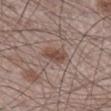<record>
  <biopsy_status>not biopsied; imaged during a skin examination</biopsy_status>
  <automated_metrics>
    <area_mm2_approx>5.0</area_mm2_approx>
    <eccentricity>0.75</eccentricity>
    <shape_asymmetry>0.2</shape_asymmetry>
    <border_irregularity_0_10>2.0</border_irregularity_0_10>
    <color_variation_0_10>2.5</color_variation_0_10>
    <nevus_likeness_0_100>70</nevus_likeness_0_100>
    <lesion_detection_confidence_0_100>100</lesion_detection_confidence_0_100>
  </automated_metrics>
  <lighting>white-light</lighting>
  <patient>
    <sex>male</sex>
    <age_approx>60</age_approx>
  </patient>
  <image>
    <source>total-body photography crop</source>
    <field_of_view_mm>15</field_of_view_mm>
  </image>
  <lesion_size>
    <long_diameter_mm_approx>3.0</long_diameter_mm_approx>
  </lesion_size>
  <site>right lower leg</site>
</record>